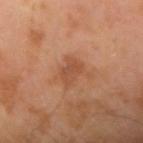Findings:
- image: ~15 mm tile from a whole-body skin photo
- site: the right upper arm
- tile lighting: cross-polarized
- subject: male, aged approximately 55